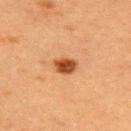Part of a total-body skin-imaging series; this lesion was reviewed on a skin check and was not flagged for biopsy. Imaged with cross-polarized lighting. A female patient roughly 55 years of age. The lesion's longest dimension is about 2.5 mm. The lesion-visualizer software estimated a within-lesion color-variation index near 3/10 and peripheral color asymmetry of about 1. The analysis additionally found a detector confidence of about 100 out of 100 that the crop contains a lesion. A region of skin cropped from a whole-body photographic capture, roughly 15 mm wide. The lesion is located on the upper back.